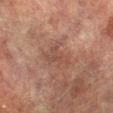Q: Is there a histopathology result?
A: total-body-photography surveillance lesion; no biopsy
Q: How large is the lesion?
A: ≈4 mm
Q: Patient demographics?
A: male, aged 73 to 77
Q: What is the anatomic site?
A: the left lower leg
Q: What kind of image is this?
A: 15 mm crop, total-body photography
Q: What did automated image analysis measure?
A: an average lesion color of about L≈39 a*≈18 b*≈23 (CIELAB) and about 5 CIELAB-L* units darker than the surrounding skin; an automated nevus-likeness rating near 0 out of 100 and a detector confidence of about 95 out of 100 that the crop contains a lesion
Q: Illumination type?
A: cross-polarized illumination